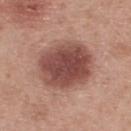Acquisition and patient details:
About 6.5 mm across. The total-body-photography lesion software estimated an eccentricity of roughly 0.55 and a symmetry-axis asymmetry near 0.1. The analysis additionally found a border-irregularity rating of about 1/10, a color-variation rating of about 4.5/10, and a peripheral color-asymmetry measure near 1.5. It also reported lesion-presence confidence of about 100/100. The lesion is located on the upper back. A 15 mm crop from a total-body photograph taken for skin-cancer surveillance. A male subject aged 63 to 67. The tile uses white-light illumination.